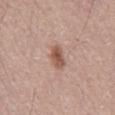Recorded during total-body skin imaging; not selected for excision or biopsy.
A male patient aged approximately 55.
A 15 mm crop from a total-body photograph taken for skin-cancer surveillance.
This is a white-light tile.